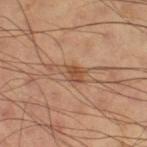  biopsy_status: not biopsied; imaged during a skin examination
  lesion_size:
    long_diameter_mm_approx: 3.0
  site: right thigh
  image:
    source: total-body photography crop
    field_of_view_mm: 15
  lighting: cross-polarized
  patient:
    sex: male
    age_approx: 60